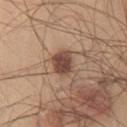This lesion was catalogued during total-body skin photography and was not selected for biopsy. Approximately 3 mm at its widest. Imaged with white-light lighting. A roughly 15 mm field-of-view crop from a total-body skin photograph. The lesion is on the chest. The subject is a male approximately 45 years of age.